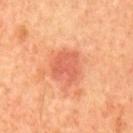{
  "biopsy_status": "not biopsied; imaged during a skin examination",
  "patient": {
    "sex": "male",
    "age_approx": 60
  },
  "site": "mid back",
  "image": {
    "source": "total-body photography crop",
    "field_of_view_mm": 15
  }
}